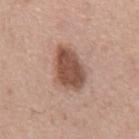No biopsy was performed on this lesion — it was imaged during a full skin examination and was not determined to be concerning. A 15 mm close-up extracted from a 3D total-body photography capture. A male subject aged around 65. From the abdomen.